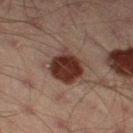notes: imaged on a skin check; not biopsied | lighting: cross-polarized illumination | TBP lesion metrics: a classifier nevus-likeness of about 95/100 and a detector confidence of about 100 out of 100 that the crop contains a lesion | diameter: ~4.5 mm (longest diameter) | acquisition: ~15 mm tile from a whole-body skin photo | patient: male, aged 43 to 47 | site: the left thigh.